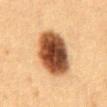{"biopsy_status": "not biopsied; imaged during a skin examination", "lesion_size": {"long_diameter_mm_approx": 6.5}, "image": {"source": "total-body photography crop", "field_of_view_mm": 15}, "site": "chest", "patient": {"sex": "male", "age_approx": 50}, "lighting": "cross-polarized"}